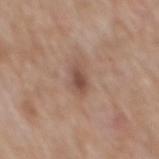Assessment: Part of a total-body skin-imaging series; this lesion was reviewed on a skin check and was not flagged for biopsy. Background: Approximately 3 mm at its widest. Captured under white-light illumination. From the mid back. The lesion-visualizer software estimated an average lesion color of about L≈49 a*≈19 b*≈26 (CIELAB), roughly 10 lightness units darker than nearby skin, and a lesion-to-skin contrast of about 7.5 (normalized; higher = more distinct). The analysis additionally found border irregularity of about 2.5 on a 0–10 scale, a color-variation rating of about 2.5/10, and a peripheral color-asymmetry measure near 1. The analysis additionally found an automated nevus-likeness rating near 25 out of 100 and lesion-presence confidence of about 100/100. Cropped from a whole-body photographic skin survey; the tile spans about 15 mm. A male patient, roughly 60 years of age.